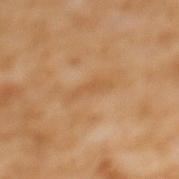Part of a total-body skin-imaging series; this lesion was reviewed on a skin check and was not flagged for biopsy. The lesion-visualizer software estimated a lesion area of about 3.5 mm², an outline eccentricity of about 0.95 (0 = round, 1 = elongated), and two-axis asymmetry of about 0.5. It also reported an automated nevus-likeness rating near 0 out of 100 and a detector confidence of about 100 out of 100 that the crop contains a lesion. Cropped from a total-body skin-imaging series; the visible field is about 15 mm. From the mid back. Imaged with cross-polarized lighting. The lesion's longest dimension is about 4 mm. The patient is a female aged around 55.